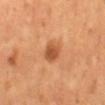On the mid back. The lesion-visualizer software estimated a lesion area of about 4.5 mm² and two-axis asymmetry of about 0.15. And it measured a border-irregularity index near 1.5/10, a within-lesion color-variation index near 2.5/10, and a peripheral color-asymmetry measure near 0.5. The software also gave a classifier nevus-likeness of about 70/100. A male subject aged around 55. A close-up tile cropped from a whole-body skin photograph, about 15 mm across. Captured under cross-polarized illumination. The lesion's longest dimension is about 2.5 mm.